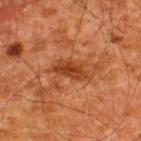Notes:
– workup — no biopsy performed (imaged during a skin exam)
– tile lighting — cross-polarized
– body site — the upper back
– image-analysis metrics — border irregularity of about 3.5 on a 0–10 scale, internal color variation of about 3 on a 0–10 scale, and a peripheral color-asymmetry measure near 1; a classifier nevus-likeness of about 0/100
– diameter — about 4.5 mm
– subject — male, aged 58 to 62
– imaging modality — ~15 mm tile from a whole-body skin photo